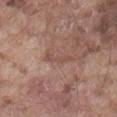notes = total-body-photography surveillance lesion; no biopsy
subject = male, aged 73–77
acquisition = ~15 mm tile from a whole-body skin photo
tile lighting = white-light illumination
location = the abdomen
lesion diameter = ~3.5 mm (longest diameter)
automated metrics = a classifier nevus-likeness of about 0/100 and a detector confidence of about 75 out of 100 that the crop contains a lesion